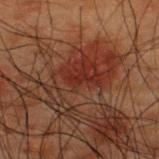Q: Was this lesion biopsied?
A: total-body-photography surveillance lesion; no biopsy
Q: How was this image acquired?
A: 15 mm crop, total-body photography
Q: What are the patient's age and sex?
A: male, aged around 50
Q: Lesion location?
A: the upper back